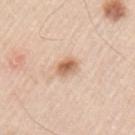workup — catalogued during a skin exam; not biopsied
location — the left upper arm
lesion diameter — ~3 mm (longest diameter)
tile lighting — white-light
imaging modality — total-body-photography crop, ~15 mm field of view
TBP lesion metrics — a normalized lesion–skin contrast near 9; a border-irregularity rating of about 1.5/10, a color-variation rating of about 4/10, and peripheral color asymmetry of about 1.5
subject — male, aged 43 to 47